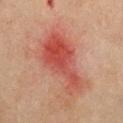Recorded during total-body skin imaging; not selected for excision or biopsy. This image is a 15 mm lesion crop taken from a total-body photograph. Captured under cross-polarized illumination. The lesion is located on the chest. Automated image analysis of the tile measured a footprint of about 24 mm², a shape eccentricity near 0.9, and a shape-asymmetry score of about 0.45 (0 = symmetric). The software also gave a lesion color around L≈45 a*≈29 b*≈28 in CIELAB. And it measured an automated nevus-likeness rating near 15 out of 100 and a lesion-detection confidence of about 100/100. A female patient aged 68 to 72.